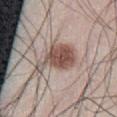Clinical impression:
Part of a total-body skin-imaging series; this lesion was reviewed on a skin check and was not flagged for biopsy.
Acquisition and patient details:
A male patient aged around 45. The recorded lesion diameter is about 5.5 mm. A roughly 15 mm field-of-view crop from a total-body skin photograph. The tile uses white-light illumination. From the abdomen.